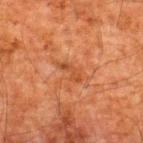| feature | finding |
|---|---|
| workup | no biopsy performed (imaged during a skin exam) |
| body site | the upper back |
| subject | male, aged approximately 60 |
| image | ~15 mm tile from a whole-body skin photo |
| automated lesion analysis | a border-irregularity rating of about 5/10, internal color variation of about 0 on a 0–10 scale, and peripheral color asymmetry of about 0 |